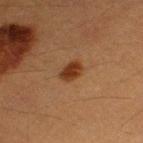Q: Was a biopsy performed?
A: total-body-photography surveillance lesion; no biopsy
Q: Lesion size?
A: about 3 mm
Q: What is the anatomic site?
A: the left thigh
Q: What lighting was used for the tile?
A: cross-polarized
Q: Patient demographics?
A: male, aged 38–42
Q: What did automated image analysis measure?
A: a footprint of about 5 mm² and a shape-asymmetry score of about 0.25 (0 = symmetric); a mean CIELAB color near L≈30 a*≈20 b*≈29, a lesion–skin lightness drop of about 10, and a lesion-to-skin contrast of about 9.5 (normalized; higher = more distinct); a within-lesion color-variation index near 2.5/10
Q: How was this image acquired?
A: total-body-photography crop, ~15 mm field of view